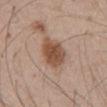The lesion was photographed on a routine skin check and not biopsied; there is no pathology result.
Imaged with white-light lighting.
A male patient, aged around 45.
Cropped from a total-body skin-imaging series; the visible field is about 15 mm.
Located on the abdomen.
Automated tile analysis of the lesion measured a lesion-detection confidence of about 100/100.